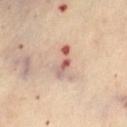Captured during whole-body skin photography for melanoma surveillance; the lesion was not biopsied. Automated tile analysis of the lesion measured an area of roughly 6.5 mm², a shape eccentricity near 0.85, and a shape-asymmetry score of about 0.45 (0 = symmetric). The analysis additionally found an average lesion color of about L≈62 a*≈20 b*≈25 (CIELAB), a lesion–skin lightness drop of about 11, and a normalized border contrast of about 7.5. A female patient roughly 60 years of age. Located on the abdomen. Captured under cross-polarized illumination. A close-up tile cropped from a whole-body skin photograph, about 15 mm across.